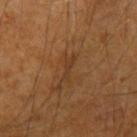• biopsy status — total-body-photography surveillance lesion; no biopsy
• patient — male, approximately 60 years of age
• anatomic site — the left upper arm
• imaging modality — ~15 mm tile from a whole-body skin photo
• illumination — cross-polarized
• diameter — ≈3.5 mm